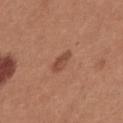Q: Is there a histopathology result?
A: imaged on a skin check; not biopsied
Q: What is the anatomic site?
A: the chest
Q: How was this image acquired?
A: total-body-photography crop, ~15 mm field of view
Q: What lighting was used for the tile?
A: white-light illumination
Q: What is the lesion's diameter?
A: ≈3 mm
Q: Who is the patient?
A: male, about 35 years old